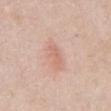Q: Was a biopsy performed?
A: total-body-photography surveillance lesion; no biopsy
Q: Lesion location?
A: the abdomen
Q: Who is the patient?
A: female, in their 60s
Q: What is the imaging modality?
A: ~15 mm tile from a whole-body skin photo
Q: Automated lesion metrics?
A: a symmetry-axis asymmetry near 0.35; an average lesion color of about L≈65 a*≈23 b*≈28 (CIELAB); border irregularity of about 4 on a 0–10 scale, a color-variation rating of about 0.5/10, and peripheral color asymmetry of about 0
Q: Illumination type?
A: white-light illumination